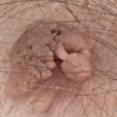Q: Was this lesion biopsied?
A: no biopsy performed (imaged during a skin exam)
Q: How was this image acquired?
A: total-body-photography crop, ~15 mm field of view
Q: What lighting was used for the tile?
A: white-light illumination
Q: What are the patient's age and sex?
A: male, aged approximately 70
Q: What is the anatomic site?
A: the chest